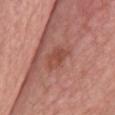notes = total-body-photography surveillance lesion; no biopsy
imaging modality = ~15 mm crop, total-body skin-cancer survey
location = the upper back
patient = female, aged 63–67
tile lighting = white-light